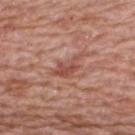Clinical impression: Imaged during a routine full-body skin examination; the lesion was not biopsied and no histopathology is available. Background: A 15 mm close-up extracted from a 3D total-body photography capture. The patient is a female aged 58–62. The lesion is on the back. Approximately 4 mm at its widest.